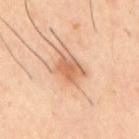The subject is a male aged 38–42. Measured at roughly 3.5 mm in maximum diameter. Imaged with cross-polarized lighting. Cropped from a whole-body photographic skin survey; the tile spans about 15 mm. The lesion is located on the mid back.